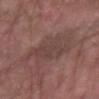<case>
<biopsy_status>not biopsied; imaged during a skin examination</biopsy_status>
<image>
  <source>total-body photography crop</source>
  <field_of_view_mm>15</field_of_view_mm>
</image>
<site>right forearm</site>
<patient>
  <sex>female</sex>
  <age_approx>70</age_approx>
</patient>
</case>